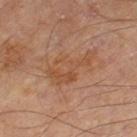biopsy status = total-body-photography surveillance lesion; no biopsy